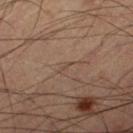<record>
  <biopsy_status>not biopsied; imaged during a skin examination</biopsy_status>
  <automated_metrics>
    <area_mm2_approx>0.5</area_mm2_approx>
    <eccentricity>0.9</eccentricity>
    <cielab_L>40</cielab_L>
    <cielab_a>14</cielab_a>
    <cielab_b>23</cielab_b>
    <vs_skin_darker_L>5.0</vs_skin_darker_L>
    <vs_skin_contrast_norm>4.5</vs_skin_contrast_norm>
    <color_variation_0_10>0.0</color_variation_0_10>
  </automated_metrics>
  <lesion_size>
    <long_diameter_mm_approx>1.0</long_diameter_mm_approx>
  </lesion_size>
  <image>
    <source>total-body photography crop</source>
    <field_of_view_mm>15</field_of_view_mm>
  </image>
  <site>right thigh</site>
  <patient>
    <sex>male</sex>
    <age_approx>60</age_approx>
  </patient>
</record>